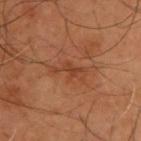Recorded during total-body skin imaging; not selected for excision or biopsy. The lesion is located on the upper back. The patient is a male in their mid-50s. About 4 mm across. Imaged with cross-polarized lighting. This image is a 15 mm lesion crop taken from a total-body photograph.